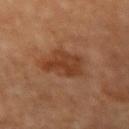The lesion was tiled from a total-body skin photograph and was not biopsied.
Captured under cross-polarized illumination.
On the right forearm.
A female subject, about 70 years old.
About 5.5 mm across.
This image is a 15 mm lesion crop taken from a total-body photograph.